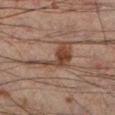Recorded during total-body skin imaging; not selected for excision or biopsy. Imaged with cross-polarized lighting. The lesion's longest dimension is about 6.5 mm. A 15 mm close-up extracted from a 3D total-body photography capture. A male subject, approximately 60 years of age. From the left lower leg.